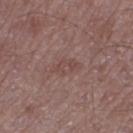notes = imaged on a skin check; not biopsied
anatomic site = the left thigh
lesion size = ≈3 mm
TBP lesion metrics = a classifier nevus-likeness of about 0/100 and lesion-presence confidence of about 100/100
patient = male, aged 48 to 52
image source = total-body-photography crop, ~15 mm field of view
lighting = white-light